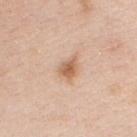{
  "image": {
    "source": "total-body photography crop",
    "field_of_view_mm": 15
  },
  "site": "chest",
  "patient": {
    "sex": "female",
    "age_approx": 45
  },
  "automated_metrics": {
    "border_irregularity_0_10": 2.5,
    "color_variation_0_10": 4.0,
    "nevus_likeness_0_100": 90,
    "lesion_detection_confidence_0_100": 100
  }
}